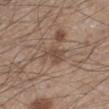| field | value |
|---|---|
| follow-up | no biopsy performed (imaged during a skin exam) |
| imaging modality | 15 mm crop, total-body photography |
| subject | male, roughly 45 years of age |
| body site | the left lower leg |
| tile lighting | white-light |